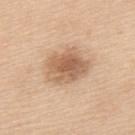Captured during whole-body skin photography for melanoma surveillance; the lesion was not biopsied. The lesion is on the upper back. A 15 mm crop from a total-body photograph taken for skin-cancer surveillance. The tile uses white-light illumination. A female patient, aged around 55. The lesion-visualizer software estimated an area of roughly 15 mm², an eccentricity of roughly 0.65, and a symmetry-axis asymmetry near 0.2. And it measured a mean CIELAB color near L≈61 a*≈19 b*≈33 and about 12 CIELAB-L* units darker than the surrounding skin. The software also gave a nevus-likeness score of about 50/100 and a detector confidence of about 100 out of 100 that the crop contains a lesion. About 5.5 mm across.